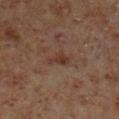This lesion was catalogued during total-body skin photography and was not selected for biopsy. This image is a 15 mm lesion crop taken from a total-body photograph. Longest diameter approximately 3 mm. This is a cross-polarized tile. From the left lower leg. The patient is a male aged 58–62.